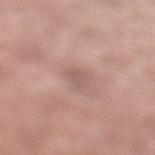Imaged during a routine full-body skin examination; the lesion was not biopsied and no histopathology is available.
A lesion tile, about 15 mm wide, cut from a 3D total-body photograph.
A male patient approximately 55 years of age.
On the left lower leg.
The tile uses white-light illumination.